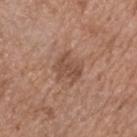The lesion was tiled from a total-body skin photograph and was not biopsied. Captured under white-light illumination. On the head or neck. An algorithmic analysis of the crop reported an area of roughly 8 mm². It also reported a normalized lesion–skin contrast near 6. Approximately 3.5 mm at its widest. A 15 mm close-up tile from a total-body photography series done for melanoma screening. A male subject approximately 50 years of age.